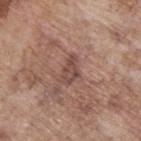From the upper back. Measured at roughly 3.5 mm in maximum diameter. A lesion tile, about 15 mm wide, cut from a 3D total-body photograph. The patient is a male aged around 70. This is a white-light tile.